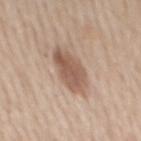The lesion was tiled from a total-body skin photograph and was not biopsied. The subject is a male aged 58 to 62. The lesion's longest dimension is about 5.5 mm. The total-body-photography lesion software estimated an automated nevus-likeness rating near 65 out of 100. From the mid back. Cropped from a total-body skin-imaging series; the visible field is about 15 mm.